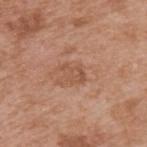Case summary:
• image source: ~15 mm tile from a whole-body skin photo
• subject: male, aged approximately 55
• lesion size: about 3 mm
• body site: the back
• tile lighting: white-light
• TBP lesion metrics: a mean CIELAB color near L≈53 a*≈22 b*≈31, a lesion–skin lightness drop of about 7, and a lesion-to-skin contrast of about 5.5 (normalized; higher = more distinct); a color-variation rating of about 2.5/10 and radial color variation of about 1; a nevus-likeness score of about 0/100 and a detector confidence of about 100 out of 100 that the crop contains a lesion